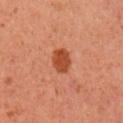On the chest. The tile uses cross-polarized illumination. A male patient aged approximately 50. A 15 mm crop from a total-body photograph taken for skin-cancer surveillance.